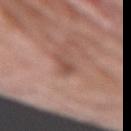This lesion was catalogued during total-body skin photography and was not selected for biopsy. This image is a 15 mm lesion crop taken from a total-body photograph. The lesion is located on the left upper arm. The subject is a female approximately 70 years of age. The recorded lesion diameter is about 2.5 mm. The lesion-visualizer software estimated a lesion area of about 3 mm² and an outline eccentricity of about 0.8 (0 = round, 1 = elongated). The software also gave a lesion color around L≈50 a*≈21 b*≈26 in CIELAB, a lesion–skin lightness drop of about 9, and a normalized border contrast of about 6.5. It also reported a border-irregularity rating of about 4.5/10 and internal color variation of about 2 on a 0–10 scale. And it measured a classifier nevus-likeness of about 0/100 and lesion-presence confidence of about 95/100.